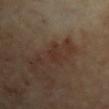The lesion was tiled from a total-body skin photograph and was not biopsied. The patient is a female roughly 65 years of age. A 15 mm crop from a total-body photograph taken for skin-cancer surveillance. From the left upper arm.